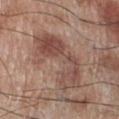Captured during whole-body skin photography for melanoma surveillance; the lesion was not biopsied. A male patient, aged around 70. Cropped from a total-body skin-imaging series; the visible field is about 15 mm. On the right lower leg.